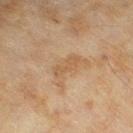Case summary:
* workup — no biopsy performed (imaged during a skin exam)
* diameter — ~3.5 mm (longest diameter)
* patient — female, approximately 60 years of age
* tile lighting — cross-polarized illumination
* image source — total-body-photography crop, ~15 mm field of view
* location — the left lower leg